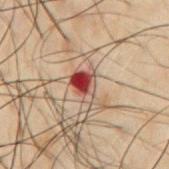- anatomic site · the front of the torso
- image source · ~15 mm tile from a whole-body skin photo
- subject · male, in their 50s